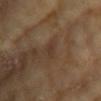The lesion was photographed on a routine skin check and not biopsied; there is no pathology result. A female subject, in their mid- to late 70s. Located on the left upper arm. This image is a 15 mm lesion crop taken from a total-body photograph. The lesion-visualizer software estimated a footprint of about 3.5 mm² and two-axis asymmetry of about 0.35. Approximately 2.5 mm at its widest.